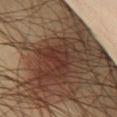Findings:
• biopsy status: catalogued during a skin exam; not biopsied
• image-analysis metrics: a footprint of about 5.5 mm², an outline eccentricity of about 0.75 (0 = round, 1 = elongated), and a shape-asymmetry score of about 0.5 (0 = symmetric); a mean CIELAB color near L≈32 a*≈21 b*≈24, a lesion–skin lightness drop of about 5, and a lesion-to-skin contrast of about 5.5 (normalized; higher = more distinct); a border-irregularity rating of about 5.5/10, a color-variation rating of about 2.5/10, and radial color variation of about 1
• patient: male, aged 48 to 52
• image source: ~15 mm crop, total-body skin-cancer survey
• anatomic site: the front of the torso
• illumination: cross-polarized
• size: about 3.5 mm